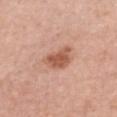The lesion was photographed on a routine skin check and not biopsied; there is no pathology result.
From the chest.
Automated image analysis of the tile measured an outline eccentricity of about 0.75 (0 = round, 1 = elongated). The software also gave a border-irregularity rating of about 3/10 and a peripheral color-asymmetry measure near 1. And it measured a classifier nevus-likeness of about 80/100 and a detector confidence of about 100 out of 100 that the crop contains a lesion.
The tile uses white-light illumination.
Longest diameter approximately 4 mm.
This image is a 15 mm lesion crop taken from a total-body photograph.
A female patient in their 60s.